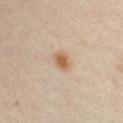The lesion was photographed on a routine skin check and not biopsied; there is no pathology result. The lesion is located on the left upper arm. A male patient aged around 35. Captured under cross-polarized illumination. A roughly 15 mm field-of-view crop from a total-body skin photograph. Approximately 2.5 mm at its widest.